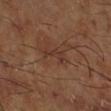Assessment: Captured during whole-body skin photography for melanoma surveillance; the lesion was not biopsied. Image and clinical context: A 15 mm close-up tile from a total-body photography series done for melanoma screening. The tile uses cross-polarized illumination. The lesion is on the right lower leg. A male subject aged around 65. The lesion-visualizer software estimated a mean CIELAB color near L≈34 a*≈18 b*≈25. The analysis additionally found a lesion-detection confidence of about 100/100. The recorded lesion diameter is about 3.5 mm.